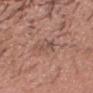Findings:
• notes · catalogued during a skin exam; not biopsied
• image source · ~15 mm crop, total-body skin-cancer survey
• site · the head or neck
• patient · male, aged 38–42
• automated metrics · a footprint of about 2.5 mm² and an outline eccentricity of about 0.9 (0 = round, 1 = elongated); an average lesion color of about L≈50 a*≈19 b*≈25 (CIELAB) and a normalized lesion–skin contrast near 5.5; a classifier nevus-likeness of about 0/100 and a lesion-detection confidence of about 85/100
• lesion diameter · ≈2.5 mm
• illumination · white-light illumination